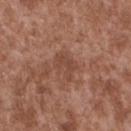follow-up = imaged on a skin check; not biopsied
subject = male, in their mid- to late 40s
image-analysis metrics = a lesion area of about 6 mm² and an eccentricity of roughly 0.7; a mean CIELAB color near L≈45 a*≈22 b*≈27 and a lesion–skin lightness drop of about 7; a nevus-likeness score of about 0/100 and lesion-presence confidence of about 100/100
imaging modality = ~15 mm tile from a whole-body skin photo
anatomic site = the upper back
illumination = white-light